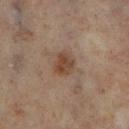notes: catalogued during a skin exam; not biopsied
subject: female, roughly 50 years of age
imaging modality: ~15 mm tile from a whole-body skin photo
anatomic site: the left lower leg